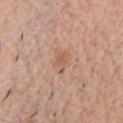  biopsy_status: not biopsied; imaged during a skin examination